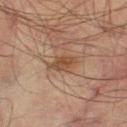{"biopsy_status": "not biopsied; imaged during a skin examination", "image": {"source": "total-body photography crop", "field_of_view_mm": 15}, "lighting": "cross-polarized", "site": "left thigh", "lesion_size": {"long_diameter_mm_approx": 3.0}, "patient": {"sex": "male", "age_approx": 75}}